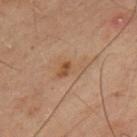This lesion was catalogued during total-body skin photography and was not selected for biopsy. This is a cross-polarized tile. A male subject aged around 60. The lesion is located on the left upper arm. This image is a 15 mm lesion crop taken from a total-body photograph. Longest diameter approximately 4 mm.